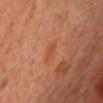Impression: No biopsy was performed on this lesion — it was imaged during a full skin examination and was not determined to be concerning. Image and clinical context: A region of skin cropped from a whole-body photographic capture, roughly 15 mm wide. Approximately 3 mm at its widest. This is a cross-polarized tile. The lesion is on the upper back. A male subject aged around 60.